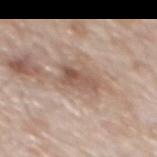Part of a total-body skin-imaging series; this lesion was reviewed on a skin check and was not flagged for biopsy. A 15 mm crop from a total-body photograph taken for skin-cancer surveillance. From the back. A male patient, in their 80s.